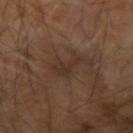Clinical impression:
Captured during whole-body skin photography for melanoma surveillance; the lesion was not biopsied.
Background:
A male patient, aged 68–72. Measured at roughly 3.5 mm in maximum diameter. A 15 mm close-up extracted from a 3D total-body photography capture. Located on the right forearm.